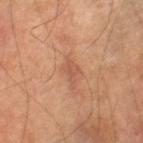This lesion was catalogued during total-body skin photography and was not selected for biopsy. A roughly 15 mm field-of-view crop from a total-body skin photograph. A male subject approximately 65 years of age. From the leg. Imaged with cross-polarized lighting. Longest diameter approximately 3 mm. The lesion-visualizer software estimated a border-irregularity index near 4.5/10 and a within-lesion color-variation index near 1/10. And it measured a detector confidence of about 100 out of 100 that the crop contains a lesion.